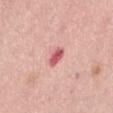Captured during whole-body skin photography for melanoma surveillance; the lesion was not biopsied.